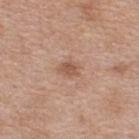notes: total-body-photography surveillance lesion; no biopsy
acquisition: ~15 mm crop, total-body skin-cancer survey
anatomic site: the upper back
image-analysis metrics: an area of roughly 4 mm² and a shape-asymmetry score of about 0.3 (0 = symmetric); a border-irregularity rating of about 3/10, a color-variation rating of about 1.5/10, and radial color variation of about 0.5
subject: female, aged around 40
tile lighting: white-light illumination
diameter: ~2.5 mm (longest diameter)